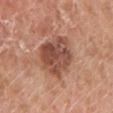No biopsy was performed on this lesion — it was imaged during a full skin examination and was not determined to be concerning. The total-body-photography lesion software estimated an average lesion color of about L≈49 a*≈23 b*≈29 (CIELAB), roughly 13 lightness units darker than nearby skin, and a normalized lesion–skin contrast near 9. And it measured lesion-presence confidence of about 100/100. The patient is a male aged around 80. Located on the left lower leg. A 15 mm close-up extracted from a 3D total-body photography capture. The recorded lesion diameter is about 5.5 mm. The tile uses white-light illumination.